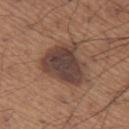The lesion was photographed on a routine skin check and not biopsied; there is no pathology result.
The lesion is on the left thigh.
The patient is a male aged 63 to 67.
Cropped from a total-body skin-imaging series; the visible field is about 15 mm.